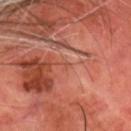Findings:
– workup · imaged on a skin check; not biopsied
– illumination · cross-polarized illumination
– location · the head or neck
– lesion size · ~1 mm (longest diameter)
– image · 15 mm crop, total-body photography
– patient · male, in their 70s